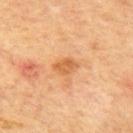{
  "biopsy_status": "not biopsied; imaged during a skin examination"
}